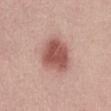{"biopsy_status": "not biopsied; imaged during a skin examination", "lighting": "white-light", "image": {"source": "total-body photography crop", "field_of_view_mm": 15}, "automated_metrics": {"area_mm2_approx": 14.0, "eccentricity": 0.3, "shape_asymmetry": 0.15, "lesion_detection_confidence_0_100": 100}, "patient": {"sex": "male", "age_approx": 40}, "lesion_size": {"long_diameter_mm_approx": 4.0}, "site": "abdomen"}